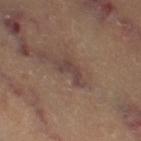Imaged during a routine full-body skin examination; the lesion was not biopsied and no histopathology is available. Located on the left thigh. Automated tile analysis of the lesion measured a lesion color around L≈40 a*≈16 b*≈19 in CIELAB, about 7 CIELAB-L* units darker than the surrounding skin, and a normalized border contrast of about 7.5. A region of skin cropped from a whole-body photographic capture, roughly 15 mm wide. Longest diameter approximately 3 mm. The tile uses cross-polarized illumination. The subject is approximately 60 years of age.